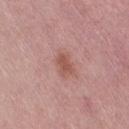Q: Who is the patient?
A: female, in their 50s
Q: How was this image acquired?
A: 15 mm crop, total-body photography
Q: Automated lesion metrics?
A: a footprint of about 4.5 mm², a shape eccentricity near 0.8, and a shape-asymmetry score of about 0.2 (0 = symmetric); internal color variation of about 2 on a 0–10 scale and peripheral color asymmetry of about 0.5
Q: What is the anatomic site?
A: the right thigh
Q: How large is the lesion?
A: ~3 mm (longest diameter)